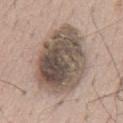– notes — catalogued during a skin exam; not biopsied
– image source — ~15 mm tile from a whole-body skin photo
– anatomic site — the chest
– size — ≈11 mm
– patient — male, in their mid- to late 60s